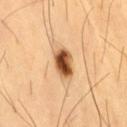Clinical impression: Recorded during total-body skin imaging; not selected for excision or biopsy. Image and clinical context: An algorithmic analysis of the crop reported a footprint of about 7 mm² and a shape-asymmetry score of about 0.15 (0 = symmetric). The analysis additionally found an average lesion color of about L≈52 a*≈24 b*≈39 (CIELAB). A 15 mm close-up extracted from a 3D total-body photography capture. The subject is a male aged approximately 60. The lesion is located on the lower back.